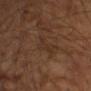Case summary:
* follow-up · no biopsy performed (imaged during a skin exam)
* subject · male, aged 63–67
* illumination · cross-polarized
* automated metrics · a mean CIELAB color near L≈29 a*≈16 b*≈25, a lesion–skin lightness drop of about 4, and a lesion-to-skin contrast of about 4.5 (normalized; higher = more distinct); a border-irregularity rating of about 5.5/10, a within-lesion color-variation index near 1.5/10, and peripheral color asymmetry of about 0.5; a nevus-likeness score of about 0/100 and a detector confidence of about 90 out of 100 that the crop contains a lesion
* image source · ~15 mm tile from a whole-body skin photo
* body site · the arm